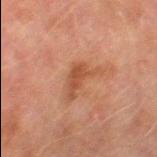| key | value |
|---|---|
| notes | catalogued during a skin exam; not biopsied |
| automated lesion analysis | an area of roughly 6 mm², an eccentricity of roughly 0.9, and a shape-asymmetry score of about 0.5 (0 = symmetric); a lesion–skin lightness drop of about 7 and a normalized border contrast of about 6.5; a border-irregularity rating of about 6.5/10, internal color variation of about 2 on a 0–10 scale, and radial color variation of about 0.5; a detector confidence of about 100 out of 100 that the crop contains a lesion |
| image | ~15 mm crop, total-body skin-cancer survey |
| site | the right thigh |
| size | about 4.5 mm |
| tile lighting | cross-polarized |
| subject | male, aged 73 to 77 |